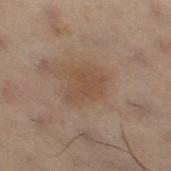Recorded during total-body skin imaging; not selected for excision or biopsy. Located on the left leg. A female patient, aged 53 to 57. About 3.5 mm across. This image is a 15 mm lesion crop taken from a total-body photograph.